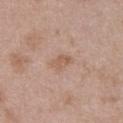{
  "biopsy_status": "not biopsied; imaged during a skin examination",
  "image": {
    "source": "total-body photography crop",
    "field_of_view_mm": 15
  },
  "patient": {
    "sex": "male",
    "age_approx": 50
  },
  "lesion_size": {
    "long_diameter_mm_approx": 2.5
  },
  "lighting": "white-light",
  "site": "front of the torso",
  "automated_metrics": {
    "eccentricity": 0.75,
    "shape_asymmetry": 0.3,
    "border_irregularity_0_10": 2.5,
    "nevus_likeness_0_100": 0,
    "lesion_detection_confidence_0_100": 100
  }
}